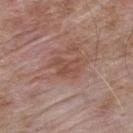Automated tile analysis of the lesion measured a lesion area of about 6 mm², a shape eccentricity near 0.75, and two-axis asymmetry of about 0.35. It also reported a lesion–skin lightness drop of about 7 and a normalized lesion–skin contrast near 6. And it measured an automated nevus-likeness rating near 0 out of 100 and lesion-presence confidence of about 100/100. The tile uses white-light illumination. The lesion's longest dimension is about 3.5 mm. Cropped from a total-body skin-imaging series; the visible field is about 15 mm. The patient is a male approximately 55 years of age. From the back.